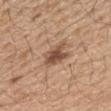Notes:
– workup — imaged on a skin check; not biopsied
– tile lighting — white-light illumination
– patient — male, aged around 70
– size — ~3.5 mm (longest diameter)
– TBP lesion metrics — a mean CIELAB color near L≈51 a*≈19 b*≈30, about 12 CIELAB-L* units darker than the surrounding skin, and a normalized border contrast of about 8.5; a classifier nevus-likeness of about 80/100 and a lesion-detection confidence of about 100/100
– site — the chest
– imaging modality — ~15 mm crop, total-body skin-cancer survey